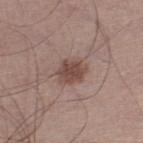Context:
The patient is a male aged around 50. Measured at roughly 3 mm in maximum diameter. Located on the right lower leg. A close-up tile cropped from a whole-body skin photograph, about 15 mm across. The tile uses white-light illumination.